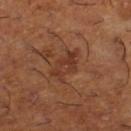Q: What lighting was used for the tile?
A: cross-polarized
Q: Lesion size?
A: ~4 mm (longest diameter)
Q: What is the anatomic site?
A: the right lower leg
Q: Who is the patient?
A: male, in their mid-60s
Q: Automated lesion metrics?
A: an average lesion color of about L≈36 a*≈23 b*≈30 (CIELAB), roughly 7 lightness units darker than nearby skin, and a lesion-to-skin contrast of about 6.5 (normalized; higher = more distinct); internal color variation of about 3.5 on a 0–10 scale and peripheral color asymmetry of about 1; an automated nevus-likeness rating near 0 out of 100 and lesion-presence confidence of about 100/100
Q: What kind of image is this?
A: ~15 mm tile from a whole-body skin photo